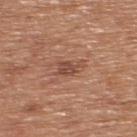<lesion>
  <biopsy_status>not biopsied; imaged during a skin examination</biopsy_status>
  <patient>
    <sex>male</sex>
    <age_approx>65</age_approx>
  </patient>
  <automated_metrics>
    <area_mm2_approx>4.0</area_mm2_approx>
    <eccentricity>0.8</eccentricity>
    <shape_asymmetry>0.35</shape_asymmetry>
    <cielab_L>48</cielab_L>
    <cielab_a>23</cielab_a>
    <cielab_b>28</cielab_b>
    <vs_skin_darker_L>9.0</vs_skin_darker_L>
    <vs_skin_contrast_norm>7.0</vs_skin_contrast_norm>
    <border_irregularity_0_10>4.0</border_irregularity_0_10>
    <color_variation_0_10>2.5</color_variation_0_10>
    <peripheral_color_asymmetry>1.0</peripheral_color_asymmetry>
    <lesion_detection_confidence_0_100>100</lesion_detection_confidence_0_100>
  </automated_metrics>
  <lighting>white-light</lighting>
  <site>upper back</site>
  <image>
    <source>total-body photography crop</source>
    <field_of_view_mm>15</field_of_view_mm>
  </image>
</lesion>